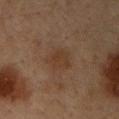The lesion was tiled from a total-body skin photograph and was not biopsied. The tile uses cross-polarized illumination. Cropped from a total-body skin-imaging series; the visible field is about 15 mm. Approximately 4 mm at its widest. The lesion is on the upper back. A male subject aged approximately 35.